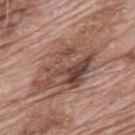• follow-up: total-body-photography surveillance lesion; no biopsy
• image: ~15 mm tile from a whole-body skin photo
• site: the mid back
• patient: male, in their 70s
• lesion diameter: ≈10 mm